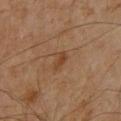This lesion was catalogued during total-body skin photography and was not selected for biopsy.
Measured at roughly 2.5 mm in maximum diameter.
From the front of the torso.
A male patient roughly 60 years of age.
Imaged with cross-polarized lighting.
A close-up tile cropped from a whole-body skin photograph, about 15 mm across.
Automated tile analysis of the lesion measured a footprint of about 3 mm², an eccentricity of roughly 0.8, and a shape-asymmetry score of about 0.3 (0 = symmetric). The software also gave an average lesion color of about L≈38 a*≈19 b*≈31 (CIELAB), a lesion–skin lightness drop of about 7, and a lesion-to-skin contrast of about 6.5 (normalized; higher = more distinct). And it measured internal color variation of about 1.5 on a 0–10 scale and radial color variation of about 0.5.